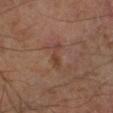The lesion was tiled from a total-body skin photograph and was not biopsied.
Captured under cross-polarized illumination.
Measured at roughly 3.5 mm in maximum diameter.
From the left lower leg.
The patient is in their mid- to late 60s.
Cropped from a total-body skin-imaging series; the visible field is about 15 mm.